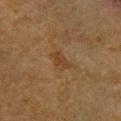Captured during whole-body skin photography for melanoma surveillance; the lesion was not biopsied. A male patient, aged 58–62. The lesion is located on the leg. Imaged with cross-polarized lighting. The lesion's longest dimension is about 2.5 mm. A roughly 15 mm field-of-view crop from a total-body skin photograph.